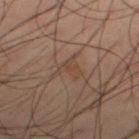Impression: Imaged during a routine full-body skin examination; the lesion was not biopsied and no histopathology is available. Image and clinical context: From the right thigh. Automated image analysis of the tile measured a footprint of about 4.5 mm², a shape eccentricity near 0.6, and a symmetry-axis asymmetry near 0.55. About 3 mm across. A male patient, aged 38 to 42. This image is a 15 mm lesion crop taken from a total-body photograph.